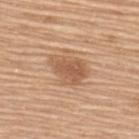The lesion was tiled from a total-body skin photograph and was not biopsied. Located on the upper back. The subject is a female approximately 60 years of age. The tile uses white-light illumination. Cropped from a total-body skin-imaging series; the visible field is about 15 mm. The recorded lesion diameter is about 4.5 mm.